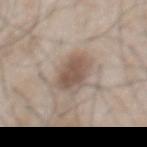| field | value |
|---|---|
| subject | male, roughly 50 years of age |
| anatomic site | the chest |
| imaging modality | ~15 mm tile from a whole-body skin photo |